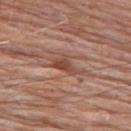{
  "biopsy_status": "not biopsied; imaged during a skin examination",
  "lesion_size": {
    "long_diameter_mm_approx": 4.0
  },
  "lighting": "white-light",
  "automated_metrics": {
    "cielab_L": 48,
    "cielab_a": 22,
    "cielab_b": 27,
    "vs_skin_darker_L": 10.0,
    "vs_skin_contrast_norm": 7.0,
    "border_irregularity_0_10": 5.5,
    "peripheral_color_asymmetry": 1.0
  },
  "patient": {
    "sex": "male",
    "age_approx": 80
  },
  "site": "upper back",
  "image": {
    "source": "total-body photography crop",
    "field_of_view_mm": 15
  }
}